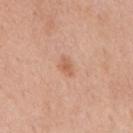* biopsy status · imaged on a skin check; not biopsied